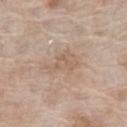Clinical impression:
Captured during whole-body skin photography for melanoma surveillance; the lesion was not biopsied.
Image and clinical context:
The patient is a female in their mid- to late 70s. Automated image analysis of the tile measured a footprint of about 8 mm², an eccentricity of roughly 0.8, and a symmetry-axis asymmetry near 0.5. And it measured a lesion color around L≈60 a*≈15 b*≈28 in CIELAB, roughly 7 lightness units darker than nearby skin, and a normalized border contrast of about 5. It also reported a classifier nevus-likeness of about 0/100 and a lesion-detection confidence of about 100/100. A lesion tile, about 15 mm wide, cut from a 3D total-body photograph. Measured at roughly 4.5 mm in maximum diameter. On the leg. Imaged with white-light lighting.